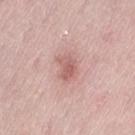No biopsy was performed on this lesion — it was imaged during a full skin examination and was not determined to be concerning. Imaged with white-light lighting. On the lower back. Cropped from a whole-body photographic skin survey; the tile spans about 15 mm. Measured at roughly 3 mm in maximum diameter. The patient is a female aged around 40.